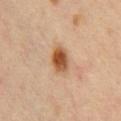notes: no biopsy performed (imaged during a skin exam)
body site: the chest
imaging modality: ~15 mm tile from a whole-body skin photo
patient: male, aged 63–67
lesion size: about 3.5 mm